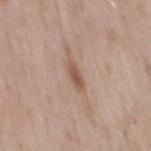Captured during whole-body skin photography for melanoma surveillance; the lesion was not biopsied. Cropped from a whole-body photographic skin survey; the tile spans about 15 mm. A male patient aged 53–57. On the mid back. Imaged with white-light lighting. The lesion's longest dimension is about 3 mm.